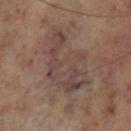| key | value |
|---|---|
| biopsy status | catalogued during a skin exam; not biopsied |
| location | the left lower leg |
| acquisition | 15 mm crop, total-body photography |
| patient | male, approximately 70 years of age |